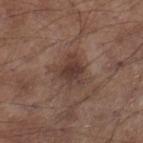Approximately 4 mm at its widest. Imaged with white-light lighting. A male patient, aged 53 to 57. A 15 mm crop from a total-body photograph taken for skin-cancer surveillance. Located on the leg.